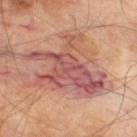The lesion was tiled from a total-body skin photograph and was not biopsied. A 15 mm close-up tile from a total-body photography series done for melanoma screening. Imaged with cross-polarized lighting. A male patient, roughly 70 years of age. Located on the right thigh. Automated tile analysis of the lesion measured a lesion color around L≈53 a*≈25 b*≈25 in CIELAB. The software also gave a border-irregularity rating of about 9/10, internal color variation of about 9.5 on a 0–10 scale, and radial color variation of about 3.5.